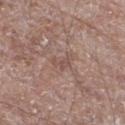workup = imaged on a skin check; not biopsied
TBP lesion metrics = roughly 7 lightness units darker than nearby skin and a normalized border contrast of about 5
tile lighting = white-light illumination
subject = male, roughly 70 years of age
body site = the right thigh
image source = 15 mm crop, total-body photography
size = about 3 mm